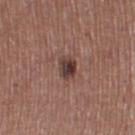Part of a total-body skin-imaging series; this lesion was reviewed on a skin check and was not flagged for biopsy.
Approximately 3 mm at its widest.
The tile uses white-light illumination.
From the leg.
A female patient aged around 55.
A region of skin cropped from a whole-body photographic capture, roughly 15 mm wide.